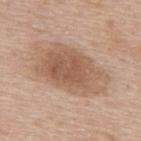The lesion was tiled from a total-body skin photograph and was not biopsied.
Imaged with white-light lighting.
A male patient, aged around 75.
Cropped from a whole-body photographic skin survey; the tile spans about 15 mm.
The lesion's longest dimension is about 8 mm.
The lesion is on the upper back.